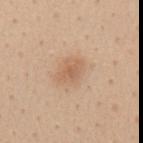  lesion_size:
    long_diameter_mm_approx: 3.0
  automated_metrics:
    eccentricity: 0.7
    shape_asymmetry: 0.25
  patient:
    sex: female
    age_approx: 45
  image:
    source: total-body photography crop
    field_of_view_mm: 15
  site: mid back